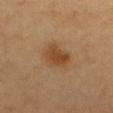Clinical impression: The lesion was photographed on a routine skin check and not biopsied; there is no pathology result. Background: This is a cross-polarized tile. The lesion-visualizer software estimated a lesion color around L≈44 a*≈19 b*≈35 in CIELAB, about 9 CIELAB-L* units darker than the surrounding skin, and a normalized border contrast of about 7.5. The lesion is located on the chest. The subject is aged around 70. Longest diameter approximately 3.5 mm. A 15 mm crop from a total-body photograph taken for skin-cancer surveillance.